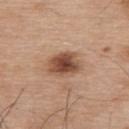This lesion was catalogued during total-body skin photography and was not selected for biopsy.
This is a white-light tile.
The subject is a male aged approximately 75.
A 15 mm close-up extracted from a 3D total-body photography capture.
Approximately 4 mm at its widest.
The lesion is on the upper back.